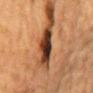Captured during whole-body skin photography for melanoma surveillance; the lesion was not biopsied. The patient is a male aged 83 or over. Measured at roughly 4.5 mm in maximum diameter. Captured under cross-polarized illumination. Located on the front of the torso. A roughly 15 mm field-of-view crop from a total-body skin photograph.